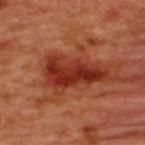Impression: This lesion was catalogued during total-body skin photography and was not selected for biopsy. Background: Longest diameter approximately 7 mm. The lesion is located on the back. A male patient roughly 50 years of age. Cropped from a total-body skin-imaging series; the visible field is about 15 mm. Automated image analysis of the tile measured a lesion area of about 18 mm², an eccentricity of roughly 0.9, and a shape-asymmetry score of about 0.4 (0 = symmetric). It also reported a lesion color around L≈34 a*≈33 b*≈33 in CIELAB, a lesion–skin lightness drop of about 13, and a lesion-to-skin contrast of about 10.5 (normalized; higher = more distinct). It also reported an automated nevus-likeness rating near 15 out of 100 and a lesion-detection confidence of about 100/100.